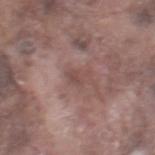Recorded during total-body skin imaging; not selected for excision or biopsy.
A male subject aged around 75.
Automated image analysis of the tile measured an average lesion color of about L≈47 a*≈19 b*≈21 (CIELAB), about 7 CIELAB-L* units darker than the surrounding skin, and a normalized lesion–skin contrast near 5.5. The software also gave a border-irregularity index near 5/10, internal color variation of about 1 on a 0–10 scale, and radial color variation of about 0.5. And it measured an automated nevus-likeness rating near 0 out of 100 and lesion-presence confidence of about 95/100.
A 15 mm crop from a total-body photograph taken for skin-cancer surveillance.
From the leg.
Longest diameter approximately 2.5 mm.
This is a white-light tile.